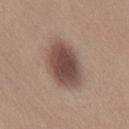workup: total-body-photography surveillance lesion; no biopsy | automated lesion analysis: a lesion area of about 18 mm², an eccentricity of roughly 0.8, and a shape-asymmetry score of about 0.15 (0 = symmetric); a lesion–skin lightness drop of about 14 and a lesion-to-skin contrast of about 10.5 (normalized; higher = more distinct); a border-irregularity rating of about 1.5/10 and peripheral color asymmetry of about 1; an automated nevus-likeness rating near 85 out of 100 and a lesion-detection confidence of about 100/100 | patient: female, in their 30s | image source: ~15 mm tile from a whole-body skin photo | location: the right thigh | lighting: white-light.